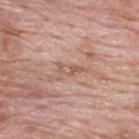Recorded during total-body skin imaging; not selected for excision or biopsy. The subject is a male aged 58–62. The lesion-visualizer software estimated a lesion area of about 3 mm² and an outline eccentricity of about 0.95 (0 = round, 1 = elongated). It also reported a lesion–skin lightness drop of about 8 and a normalized border contrast of about 5.5. A roughly 15 mm field-of-view crop from a total-body skin photograph. The recorded lesion diameter is about 3 mm. The lesion is on the back. This is a white-light tile.